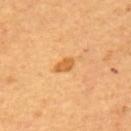follow-up: total-body-photography surveillance lesion; no biopsy | lesion diameter: ~2.5 mm (longest diameter) | automated metrics: a lesion color around L≈63 a*≈26 b*≈49 in CIELAB and about 11 CIELAB-L* units darker than the surrounding skin; a border-irregularity index near 2/10 and a within-lesion color-variation index near 1.5/10 | lighting: cross-polarized illumination | patient: male, about 65 years old | location: the upper back | imaging modality: 15 mm crop, total-body photography.